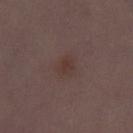Assessment:
Imaged during a routine full-body skin examination; the lesion was not biopsied and no histopathology is available.
Background:
A female subject, aged 28 to 32. On the leg. A 15 mm close-up tile from a total-body photography series done for melanoma screening.